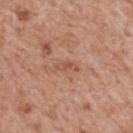Findings:
– workup — total-body-photography surveillance lesion; no biopsy
– subject — male, aged approximately 65
– anatomic site — the back
– image source — ~15 mm tile from a whole-body skin photo
– lighting — white-light